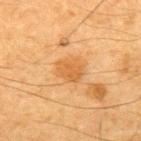Assessment: The lesion was photographed on a routine skin check and not biopsied; there is no pathology result. Context: A male subject roughly 65 years of age. A 15 mm close-up extracted from a 3D total-body photography capture. The lesion is on the back.